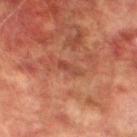Part of a total-body skin-imaging series; this lesion was reviewed on a skin check and was not flagged for biopsy. A 15 mm close-up tile from a total-body photography series done for melanoma screening. Imaged with cross-polarized lighting. A male patient aged around 75. The recorded lesion diameter is about 3 mm. The lesion is on the leg.